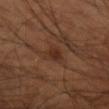No biopsy was performed on this lesion — it was imaged during a full skin examination and was not determined to be concerning.
Captured under cross-polarized illumination.
Cropped from a whole-body photographic skin survey; the tile spans about 15 mm.
The lesion is located on the right forearm.
The subject is a male aged 53 to 57.